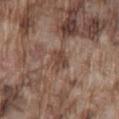{"biopsy_status": "not biopsied; imaged during a skin examination", "image": {"source": "total-body photography crop", "field_of_view_mm": 15}, "patient": {"sex": "male", "age_approx": 75}, "lighting": "white-light", "lesion_size": {"long_diameter_mm_approx": 3.0}, "site": "lower back", "automated_metrics": {"cielab_L": 43, "cielab_a": 17, "cielab_b": 25, "vs_skin_darker_L": 9.0, "vs_skin_contrast_norm": 7.5, "nevus_likeness_0_100": 0, "lesion_detection_confidence_0_100": 75}}